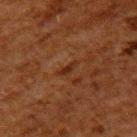biopsy status: total-body-photography surveillance lesion; no biopsy | image-analysis metrics: a footprint of about 2 mm², an outline eccentricity of about 0.95 (0 = round, 1 = elongated), and a shape-asymmetry score of about 0.5 (0 = symmetric); a mean CIELAB color near L≈22 a*≈19 b*≈26 and a lesion–skin lightness drop of about 6; a classifier nevus-likeness of about 0/100 | subject: male, aged 58 to 62 | site: the back | image source: ~15 mm crop, total-body skin-cancer survey.